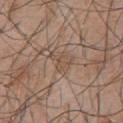Captured during whole-body skin photography for melanoma surveillance; the lesion was not biopsied. A roughly 15 mm field-of-view crop from a total-body skin photograph. The lesion is located on the front of the torso. A male subject, aged 43 to 47.